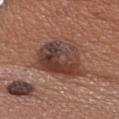Recorded during total-body skin imaging; not selected for excision or biopsy. The recorded lesion diameter is about 7 mm. The lesion is on the head or neck. The total-body-photography lesion software estimated a lesion color around L≈40 a*≈20 b*≈24 in CIELAB and a lesion-to-skin contrast of about 10 (normalized; higher = more distinct). A close-up tile cropped from a whole-body skin photograph, about 15 mm across. A male subject approximately 35 years of age.